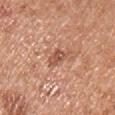Q: Is there a histopathology result?
A: imaged on a skin check; not biopsied
Q: Automated lesion metrics?
A: an eccentricity of roughly 0.85; about 10 CIELAB-L* units darker than the surrounding skin and a normalized lesion–skin contrast near 7; a nevus-likeness score of about 5/100 and lesion-presence confidence of about 100/100
Q: What lighting was used for the tile?
A: white-light
Q: What is the anatomic site?
A: the front of the torso
Q: What is the imaging modality?
A: total-body-photography crop, ~15 mm field of view
Q: Patient demographics?
A: male, roughly 30 years of age